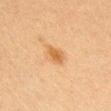notes = no biopsy performed (imaged during a skin exam); lighting = cross-polarized illumination; lesion size = ≈3 mm; image source = ~15 mm tile from a whole-body skin photo; subject = female, roughly 20 years of age; anatomic site = the head or neck.